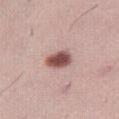workup: catalogued during a skin exam; not biopsied
patient: female, in their mid- to late 20s
image source: ~15 mm crop, total-body skin-cancer survey
location: the right thigh
automated lesion analysis: a lesion area of about 6.5 mm², an outline eccentricity of about 0.7 (0 = round, 1 = elongated), and a shape-asymmetry score of about 0.2 (0 = symmetric); a border-irregularity rating of about 2/10, internal color variation of about 4.5 on a 0–10 scale, and radial color variation of about 1.5
illumination: white-light